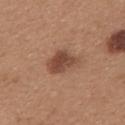Clinical impression:
Captured during whole-body skin photography for melanoma surveillance; the lesion was not biopsied.
Clinical summary:
On the back. A lesion tile, about 15 mm wide, cut from a 3D total-body photograph. The lesion-visualizer software estimated about 11 CIELAB-L* units darker than the surrounding skin and a lesion-to-skin contrast of about 8.5 (normalized; higher = more distinct). It also reported internal color variation of about 4 on a 0–10 scale and a peripheral color-asymmetry measure near 1. The software also gave an automated nevus-likeness rating near 70 out of 100 and a lesion-detection confidence of about 100/100. Imaged with white-light lighting. Longest diameter approximately 4 mm. A female patient approximately 30 years of age.